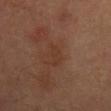Recorded during total-body skin imaging; not selected for excision or biopsy.
Automated image analysis of the tile measured an average lesion color of about L≈33 a*≈19 b*≈27 (CIELAB), a lesion–skin lightness drop of about 5, and a normalized lesion–skin contrast near 5.5. The analysis additionally found a color-variation rating of about 0/10 and a peripheral color-asymmetry measure near 0. The analysis additionally found an automated nevus-likeness rating near 0 out of 100.
About 3.5 mm across.
A lesion tile, about 15 mm wide, cut from a 3D total-body photograph.
On the mid back.
Captured under cross-polarized illumination.
The subject is a male roughly 70 years of age.